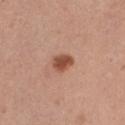| key | value |
|---|---|
| workup | total-body-photography surveillance lesion; no biopsy |
| diameter | ~2.5 mm (longest diameter) |
| location | the left thigh |
| illumination | white-light illumination |
| automated lesion analysis | a shape eccentricity near 0.7 and two-axis asymmetry of about 0.15; a mean CIELAB color near L≈50 a*≈24 b*≈30, about 14 CIELAB-L* units darker than the surrounding skin, and a normalized lesion–skin contrast near 9.5; a border-irregularity index near 1.5/10, a color-variation rating of about 2.5/10, and radial color variation of about 1 |
| subject | female, about 30 years old |
| image source | ~15 mm crop, total-body skin-cancer survey |